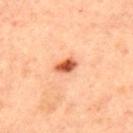Q: Was a biopsy performed?
A: no biopsy performed (imaged during a skin exam)
Q: Who is the patient?
A: female, about 45 years old
Q: What kind of image is this?
A: 15 mm crop, total-body photography
Q: What is the anatomic site?
A: the back